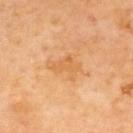Q: What is the imaging modality?
A: ~15 mm crop, total-body skin-cancer survey
Q: What is the lesion's diameter?
A: ~4 mm (longest diameter)
Q: Illumination type?
A: cross-polarized
Q: Automated lesion metrics?
A: a normalized border contrast of about 5; a border-irregularity index near 5.5/10 and radial color variation of about 0.5; an automated nevus-likeness rating near 0 out of 100 and a detector confidence of about 100 out of 100 that the crop contains a lesion
Q: Patient demographics?
A: male, aged 68 to 72
Q: Lesion location?
A: the upper back